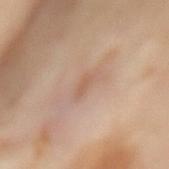No biopsy was performed on this lesion — it was imaged during a full skin examination and was not determined to be concerning. This is a cross-polarized tile. This image is a 15 mm lesion crop taken from a total-body photograph. About 2.5 mm across. A female patient, approximately 55 years of age. An algorithmic analysis of the crop reported a border-irregularity rating of about 4.5/10, internal color variation of about 0 on a 0–10 scale, and a peripheral color-asymmetry measure near 0. And it measured a nevus-likeness score of about 0/100 and lesion-presence confidence of about 100/100. The lesion is located on the mid back.